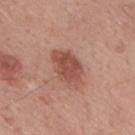Imaged during a routine full-body skin examination; the lesion was not biopsied and no histopathology is available.
The lesion is located on the mid back.
The patient is a male aged around 50.
A 15 mm close-up tile from a total-body photography series done for melanoma screening.